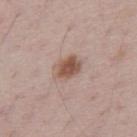Part of a total-body skin-imaging series; this lesion was reviewed on a skin check and was not flagged for biopsy. Cropped from a total-body skin-imaging series; the visible field is about 15 mm. Measured at roughly 3.5 mm in maximum diameter. An algorithmic analysis of the crop reported an area of roughly 7.5 mm², an outline eccentricity of about 0.7 (0 = round, 1 = elongated), and a shape-asymmetry score of about 0.15 (0 = symmetric). The software also gave an average lesion color of about L≈54 a*≈18 b*≈26 (CIELAB) and a lesion-to-skin contrast of about 8.5 (normalized; higher = more distinct). On the mid back. The subject is a male in their 70s. The tile uses white-light illumination.